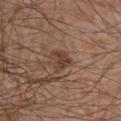No biopsy was performed on this lesion — it was imaged during a full skin examination and was not determined to be concerning. From the chest. A lesion tile, about 15 mm wide, cut from a 3D total-body photograph. A male patient in their mid-60s.